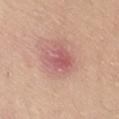This lesion was catalogued during total-body skin photography and was not selected for biopsy.
This image is a 15 mm lesion crop taken from a total-body photograph.
From the right thigh.
The subject is a female aged around 40.
Captured under white-light illumination.